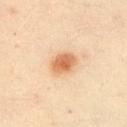Impression:
Captured during whole-body skin photography for melanoma surveillance; the lesion was not biopsied.
Clinical summary:
A close-up tile cropped from a whole-body skin photograph, about 15 mm across. From the front of the torso. A male subject aged 48 to 52.